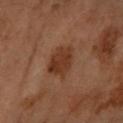Impression: Captured during whole-body skin photography for melanoma surveillance; the lesion was not biopsied. Image and clinical context: A female subject roughly 60 years of age. Captured under cross-polarized illumination. Located on the left forearm. Cropped from a total-body skin-imaging series; the visible field is about 15 mm. Approximately 4 mm at its widest.